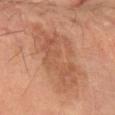biopsy status: total-body-photography surveillance lesion; no biopsy
image source: total-body-photography crop, ~15 mm field of view
anatomic site: the left forearm
patient: male, about 70 years old